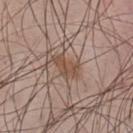notes = imaged on a skin check; not biopsied
location = the back
acquisition = 15 mm crop, total-body photography
illumination = white-light
lesion diameter = about 4 mm
subject = male, aged approximately 45